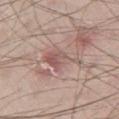| feature | finding |
|---|---|
| notes | catalogued during a skin exam; not biopsied |
| patient | male, approximately 30 years of age |
| acquisition | total-body-photography crop, ~15 mm field of view |
| site | the right thigh |